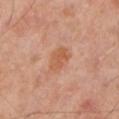Recorded during total-body skin imaging; not selected for excision or biopsy.
A male subject, aged approximately 50.
Cropped from a whole-body photographic skin survey; the tile spans about 15 mm.
This is a cross-polarized tile.
An algorithmic analysis of the crop reported an area of roughly 6 mm², an eccentricity of roughly 0.75, and a symmetry-axis asymmetry near 0.2. The analysis additionally found a lesion color around L≈56 a*≈24 b*≈34 in CIELAB. It also reported radial color variation of about 1. The software also gave a detector confidence of about 100 out of 100 that the crop contains a lesion.
The recorded lesion diameter is about 3.5 mm.
From the leg.